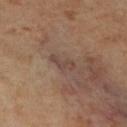Imaged during a routine full-body skin examination; the lesion was not biopsied and no histopathology is available. Captured under cross-polarized illumination. The lesion-visualizer software estimated a shape eccentricity near 0.9 and a symmetry-axis asymmetry near 0.5. It also reported a lesion color around L≈45 a*≈17 b*≈23 in CIELAB, a lesion–skin lightness drop of about 7, and a normalized lesion–skin contrast near 6. A 15 mm close-up tile from a total-body photography series done for melanoma screening. The recorded lesion diameter is about 3 mm. From the right lower leg. A female patient aged 48–52.